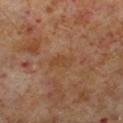Captured under cross-polarized illumination. Longest diameter approximately 3 mm. A male patient, aged 58 to 62. From the right lower leg. Cropped from a whole-body photographic skin survey; the tile spans about 15 mm.